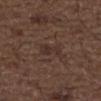The lesion was tiled from a total-body skin photograph and was not biopsied.
Captured under white-light illumination.
The lesion's longest dimension is about 3 mm.
On the right forearm.
The total-body-photography lesion software estimated a lesion area of about 4.5 mm², a shape eccentricity near 0.8, and two-axis asymmetry of about 0.4.
A close-up tile cropped from a whole-body skin photograph, about 15 mm across.
A male subject about 50 years old.